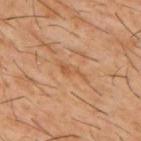<case>
<biopsy_status>not biopsied; imaged during a skin examination</biopsy_status>
<patient>
  <sex>male</sex>
  <age_approx>60</age_approx>
</patient>
<automated_metrics>
  <area_mm2_approx>3.5</area_mm2_approx>
  <eccentricity>0.95</eccentricity>
  <shape_asymmetry>0.45</shape_asymmetry>
  <nevus_likeness_0_100>0</nevus_likeness_0_100>
  <lesion_detection_confidence_0_100>70</lesion_detection_confidence_0_100>
</automated_metrics>
<lesion_size>
  <long_diameter_mm_approx>4.0</long_diameter_mm_approx>
</lesion_size>
<image>
  <source>total-body photography crop</source>
  <field_of_view_mm>15</field_of_view_mm>
</image>
<lighting>cross-polarized</lighting>
<site>upper back</site>
</case>